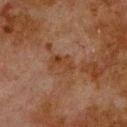No biopsy was performed on this lesion — it was imaged during a full skin examination and was not determined to be concerning. The tile uses cross-polarized illumination. The subject is a male approximately 80 years of age. Longest diameter approximately 3 mm. This image is a 15 mm lesion crop taken from a total-body photograph. The total-body-photography lesion software estimated a footprint of about 4.5 mm² and an eccentricity of roughly 0.85. The analysis additionally found a border-irregularity rating of about 3.5/10, a color-variation rating of about 3/10, and a peripheral color-asymmetry measure near 1. The lesion is located on the upper back.